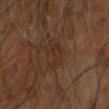Assessment: The lesion was photographed on a routine skin check and not biopsied; there is no pathology result. Background: Located on the left arm. The tile uses cross-polarized illumination. The patient is a male aged approximately 60. A close-up tile cropped from a whole-body skin photograph, about 15 mm across. The recorded lesion diameter is about 4 mm.